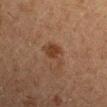The lesion was tiled from a total-body skin photograph and was not biopsied. A 15 mm close-up tile from a total-body photography series done for melanoma screening. The lesion is located on the right upper arm. A male patient, aged approximately 50.